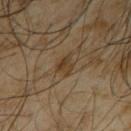{"biopsy_status": "not biopsied; imaged during a skin examination", "patient": {"sex": "male", "age_approx": 65}, "image": {"source": "total-body photography crop", "field_of_view_mm": 15}, "automated_metrics": {"area_mm2_approx": 3.5, "eccentricity": 0.7, "shape_asymmetry": 0.35, "cielab_L": 35, "cielab_a": 13, "cielab_b": 29, "vs_skin_darker_L": 8.0, "vs_skin_contrast_norm": 8.0, "border_irregularity_0_10": 3.5, "color_variation_0_10": 3.0, "nevus_likeness_0_100": 25, "lesion_detection_confidence_0_100": 100}, "lesion_size": {"long_diameter_mm_approx": 2.5}, "lighting": "cross-polarized", "site": "mid back"}